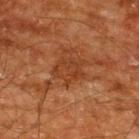{"site": "upper back", "patient": {"sex": "male", "age_approx": 60}, "image": {"source": "total-body photography crop", "field_of_view_mm": 15}}